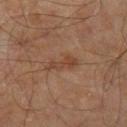image source — total-body-photography crop, ~15 mm field of view
body site — the leg
patient — male, roughly 60 years of age
TBP lesion metrics — a lesion area of about 4.5 mm² and a shape-asymmetry score of about 0.45 (0 = symmetric); a border-irregularity index near 5/10; a nevus-likeness score of about 0/100 and a detector confidence of about 100 out of 100 that the crop contains a lesion
lesion diameter — about 4 mm
tile lighting — cross-polarized illumination